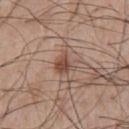biopsy status: total-body-photography surveillance lesion; no biopsy | subject: male, in their mid- to late 60s | image source: ~15 mm tile from a whole-body skin photo | anatomic site: the chest.